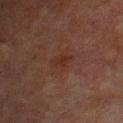biopsy status — no biopsy performed (imaged during a skin exam); subject — male, aged approximately 65; body site — the upper back; imaging modality — ~15 mm crop, total-body skin-cancer survey.